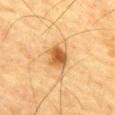notes — no biopsy performed (imaged during a skin exam)
image source — 15 mm crop, total-body photography
TBP lesion metrics — an area of roughly 6.5 mm², an outline eccentricity of about 0.45 (0 = round, 1 = elongated), and a symmetry-axis asymmetry near 0.3; a mean CIELAB color near L≈52 a*≈21 b*≈41, roughly 13 lightness units darker than nearby skin, and a normalized lesion–skin contrast near 9.5
site — the chest
subject — male, aged 83 or older
size — ≈3 mm